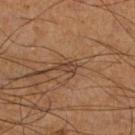Clinical impression: No biopsy was performed on this lesion — it was imaged during a full skin examination and was not determined to be concerning. Image and clinical context: The lesion's longest dimension is about 2.5 mm. A male patient, aged approximately 60. Automated tile analysis of the lesion measured an area of roughly 3 mm² and a shape eccentricity near 0.85. The analysis additionally found roughly 8 lightness units darker than nearby skin and a normalized lesion–skin contrast near 7. It also reported a border-irregularity rating of about 4/10 and peripheral color asymmetry of about 0. It also reported a nevus-likeness score of about 0/100 and a lesion-detection confidence of about 55/100. Located on the leg. A 15 mm close-up extracted from a 3D total-body photography capture.